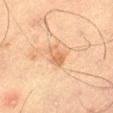Clinical summary: A close-up tile cropped from a whole-body skin photograph, about 15 mm across. The recorded lesion diameter is about 3.5 mm. Located on the leg. A male patient aged 68 to 72. Captured under cross-polarized illumination.